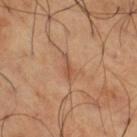| key | value |
|---|---|
| workup | no biopsy performed (imaged during a skin exam) |
| patient | male, in their mid-60s |
| site | the right thigh |
| acquisition | ~15 mm tile from a whole-body skin photo |
| lesion size | ~3 mm (longest diameter) |
| tile lighting | cross-polarized illumination |